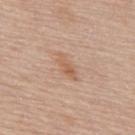Q: Was a biopsy performed?
A: catalogued during a skin exam; not biopsied
Q: How was this image acquired?
A: ~15 mm crop, total-body skin-cancer survey
Q: Lesion location?
A: the back
Q: Automated lesion metrics?
A: an automated nevus-likeness rating near 0 out of 100 and lesion-presence confidence of about 100/100
Q: Who is the patient?
A: male, aged 78–82
Q: What lighting was used for the tile?
A: white-light
Q: How large is the lesion?
A: ≈3.5 mm